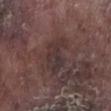Assessment: This lesion was catalogued during total-body skin photography and was not selected for biopsy. Background: From the left lower leg. The patient is a male approximately 75 years of age. Cropped from a whole-body photographic skin survey; the tile spans about 15 mm.